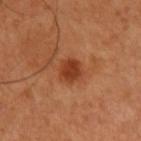Part of a total-body skin-imaging series; this lesion was reviewed on a skin check and was not flagged for biopsy.
Imaged with cross-polarized lighting.
From the right upper arm.
A male patient, roughly 50 years of age.
Approximately 3 mm at its widest.
A region of skin cropped from a whole-body photographic capture, roughly 15 mm wide.